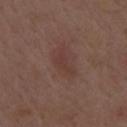Captured during whole-body skin photography for melanoma surveillance; the lesion was not biopsied.
Cropped from a whole-body photographic skin survey; the tile spans about 15 mm.
A male subject roughly 70 years of age.
The lesion is located on the mid back.